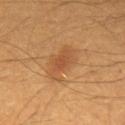Assessment: Captured during whole-body skin photography for melanoma surveillance; the lesion was not biopsied. Clinical summary: The lesion is on the mid back. A region of skin cropped from a whole-body photographic capture, roughly 15 mm wide. Automated image analysis of the tile measured a footprint of about 12 mm², an eccentricity of roughly 0.8, and a shape-asymmetry score of about 0.25 (0 = symmetric). It also reported a lesion–skin lightness drop of about 7 and a normalized lesion–skin contrast near 5.5. And it measured a classifier nevus-likeness of about 90/100. A male subject, aged 58–62. The lesion's longest dimension is about 5 mm. Captured under cross-polarized illumination.